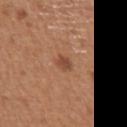Recorded during total-body skin imaging; not selected for excision or biopsy.
A 15 mm crop from a total-body photograph taken for skin-cancer surveillance.
The patient is a male aged approximately 55.
The total-body-photography lesion software estimated a normalized border contrast of about 6. And it measured border irregularity of about 1.5 on a 0–10 scale, internal color variation of about 3 on a 0–10 scale, and radial color variation of about 1.
On the chest.
Measured at roughly 2.5 mm in maximum diameter.